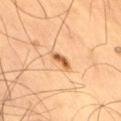workup: total-body-photography surveillance lesion; no biopsy | body site: the right thigh | image: ~15 mm tile from a whole-body skin photo | lesion diameter: ~3 mm (longest diameter) | illumination: cross-polarized illumination | patient: male, aged approximately 60 | automated lesion analysis: an outline eccentricity of about 0.85 (0 = round, 1 = elongated); a border-irregularity index near 4/10, internal color variation of about 3 on a 0–10 scale, and radial color variation of about 1.